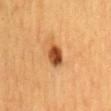Notes:
- notes — catalogued during a skin exam; not biopsied
- diameter — ~3 mm (longest diameter)
- image — ~15 mm crop, total-body skin-cancer survey
- patient — female, approximately 55 years of age
- anatomic site — the mid back
- lighting — cross-polarized illumination